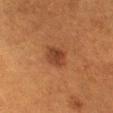Clinical impression: Recorded during total-body skin imaging; not selected for excision or biopsy. Background: Captured under cross-polarized illumination. A lesion tile, about 15 mm wide, cut from a 3D total-body photograph. On the chest. Automated image analysis of the tile measured a footprint of about 6 mm², an outline eccentricity of about 0.65 (0 = round, 1 = elongated), and two-axis asymmetry of about 0.2. It also reported a mean CIELAB color near L≈34 a*≈21 b*≈29. The subject is a female about 40 years old.